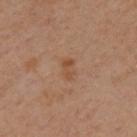No biopsy was performed on this lesion — it was imaged during a full skin examination and was not determined to be concerning. The subject is a male roughly 45 years of age. A 15 mm close-up extracted from a 3D total-body photography capture. The lesion is located on the upper back.